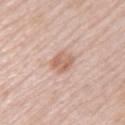{"biopsy_status": "not biopsied; imaged during a skin examination"}